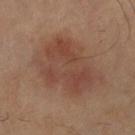{"biopsy_status": "not biopsied; imaged during a skin examination", "lesion_size": {"long_diameter_mm_approx": 7.5}, "site": "right forearm", "patient": {"sex": "male", "age_approx": 70}, "automated_metrics": {"area_mm2_approx": 34.0, "shape_asymmetry": 0.25, "cielab_L": 41, "cielab_a": 19, "cielab_b": 25, "vs_skin_darker_L": 7.0, "vs_skin_contrast_norm": 6.5, "peripheral_color_asymmetry": 1.0, "lesion_detection_confidence_0_100": 100}, "image": {"source": "total-body photography crop", "field_of_view_mm": 15}, "lighting": "cross-polarized"}